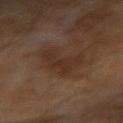{"biopsy_status": "not biopsied; imaged during a skin examination", "lighting": "cross-polarized", "image": {"source": "total-body photography crop", "field_of_view_mm": 15}, "lesion_size": {"long_diameter_mm_approx": 5.5}, "site": "right forearm", "automated_metrics": {"area_mm2_approx": 9.0, "shape_asymmetry": 0.5, "cielab_L": 29, "cielab_a": 16, "cielab_b": 24, "vs_skin_darker_L": 6.0, "vs_skin_contrast_norm": 6.0, "border_irregularity_0_10": 7.0, "color_variation_0_10": 2.0, "nevus_likeness_0_100": 0, "lesion_detection_confidence_0_100": 100}, "patient": {"sex": "male", "age_approx": 65}}